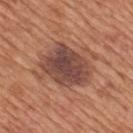The lesion was tiled from a total-body skin photograph and was not biopsied.
A male subject, aged approximately 65.
On the mid back.
A roughly 15 mm field-of-view crop from a total-body skin photograph.